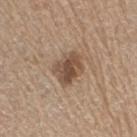Assessment: The lesion was tiled from a total-body skin photograph and was not biopsied.